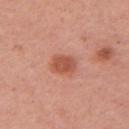• follow-up · total-body-photography surveillance lesion; no biopsy
• tile lighting · white-light illumination
• image · ~15 mm crop, total-body skin-cancer survey
• subject · female, aged 33–37
• body site · the left upper arm
• TBP lesion metrics · a lesion area of about 6.5 mm², an outline eccentricity of about 0.55 (0 = round, 1 = elongated), and a shape-asymmetry score of about 0.25 (0 = symmetric); an automated nevus-likeness rating near 95 out of 100